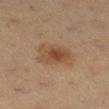The lesion is on the right lower leg. The patient is a female aged approximately 40. Captured under cross-polarized illumination. The lesion's longest dimension is about 4 mm. A 15 mm crop from a total-body photograph taken for skin-cancer surveillance.